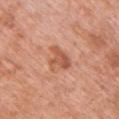  biopsy_status: not biopsied; imaged during a skin examination
  lighting: white-light
  patient:
    sex: female
    age_approx: 50
  image:
    source: total-body photography crop
    field_of_view_mm: 15
  site: chest
  automated_metrics:
    cielab_L: 56
    cielab_a: 26
    cielab_b: 33
    vs_skin_contrast_norm: 7.0
    border_irregularity_0_10: 5.5
    peripheral_color_asymmetry: 0.5
    nevus_likeness_0_100: 20
    lesion_detection_confidence_0_100: 100
  lesion_size:
    long_diameter_mm_approx: 3.5